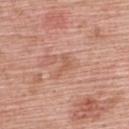{
  "biopsy_status": "not biopsied; imaged during a skin examination",
  "image": {
    "source": "total-body photography crop",
    "field_of_view_mm": 15
  },
  "site": "upper back",
  "patient": {
    "sex": "male",
    "age_approx": 60
  },
  "lighting": "white-light",
  "lesion_size": {
    "long_diameter_mm_approx": 2.5
  }
}